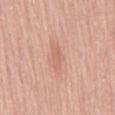Notes:
* biopsy status — catalogued during a skin exam; not biopsied
* patient — male, in their mid-60s
* illumination — white-light
* TBP lesion metrics — a footprint of about 4.5 mm², a shape eccentricity near 0.9, and a symmetry-axis asymmetry near 0.35; a lesion color around L≈64 a*≈22 b*≈30 in CIELAB, roughly 7 lightness units darker than nearby skin, and a normalized border contrast of about 4.5; a nevus-likeness score of about 0/100 and lesion-presence confidence of about 100/100
* image — total-body-photography crop, ~15 mm field of view
* lesion diameter — ≈4 mm
* anatomic site — the abdomen